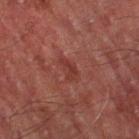<tbp_lesion>
  <biopsy_status>not biopsied; imaged during a skin examination</biopsy_status>
  <image>
    <source>total-body photography crop</source>
    <field_of_view_mm>15</field_of_view_mm>
  </image>
  <lesion_size>
    <long_diameter_mm_approx>3.0</long_diameter_mm_approx>
  </lesion_size>
  <automated_metrics>
    <nevus_likeness_0_100>0</nevus_likeness_0_100>
    <lesion_detection_confidence_0_100>100</lesion_detection_confidence_0_100>
  </automated_metrics>
  <patient>
    <sex>male</sex>
    <age_approx>70</age_approx>
  </patient>
  <lighting>cross-polarized</lighting>
  <site>left thigh</site>
</tbp_lesion>